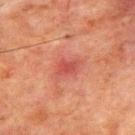This lesion was catalogued during total-body skin photography and was not selected for biopsy. A lesion tile, about 15 mm wide, cut from a 3D total-body photograph. A male patient, in their mid- to late 60s. Approximately 3.5 mm at its widest. The lesion-visualizer software estimated a mean CIELAB color near L≈42 a*≈29 b*≈26, roughly 7 lightness units darker than nearby skin, and a normalized border contrast of about 6. It also reported border irregularity of about 3.5 on a 0–10 scale, internal color variation of about 2.5 on a 0–10 scale, and radial color variation of about 1. The analysis additionally found a nevus-likeness score of about 0/100. Imaged with cross-polarized lighting. The lesion is located on the upper back.